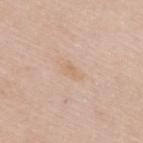Imaged during a routine full-body skin examination; the lesion was not biopsied and no histopathology is available.
The lesion's longest dimension is about 2.5 mm.
A female patient, approximately 50 years of age.
A region of skin cropped from a whole-body photographic capture, roughly 15 mm wide.
From the upper back.
Imaged with white-light lighting.